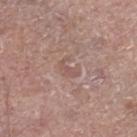Image and clinical context: A male subject, aged approximately 55. The recorded lesion diameter is about 2.5 mm. A 15 mm crop from a total-body photograph taken for skin-cancer surveillance. The lesion is on the right lower leg.